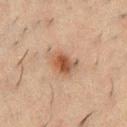Case summary:
• patient: male, about 50 years old
• illumination: cross-polarized
• imaging modality: 15 mm crop, total-body photography
• lesion size: about 3.5 mm
• TBP lesion metrics: a border-irregularity index near 2/10, a within-lesion color-variation index near 5/10, and peripheral color asymmetry of about 1.5; a classifier nevus-likeness of about 95/100 and lesion-presence confidence of about 100/100
• site: the chest